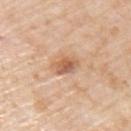follow-up=imaged on a skin check; not biopsied | body site=the left upper arm | tile lighting=white-light illumination | acquisition=~15 mm tile from a whole-body skin photo | patient=male, about 75 years old.